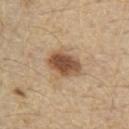Recorded during total-body skin imaging; not selected for excision or biopsy. The subject is a male roughly 70 years of age. A 15 mm close-up tile from a total-body photography series done for melanoma screening. Located on the chest. This is a white-light tile. Longest diameter approximately 4 mm.